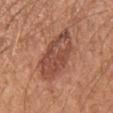Part of a total-body skin-imaging series; this lesion was reviewed on a skin check and was not flagged for biopsy. A male patient, about 55 years old. Imaged with white-light lighting. Located on the right upper arm. Longest diameter approximately 6 mm. Cropped from a total-body skin-imaging series; the visible field is about 15 mm.